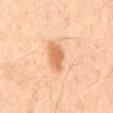  biopsy_status: not biopsied; imaged during a skin examination
  patient:
    sex: male
    age_approx: 45
  image:
    source: total-body photography crop
    field_of_view_mm: 15
  lesion_size:
    long_diameter_mm_approx: 3.5
  site: mid back
  lighting: cross-polarized